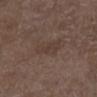Assessment:
Imaged during a routine full-body skin examination; the lesion was not biopsied and no histopathology is available.
Context:
The lesion is located on the leg. A male subject, roughly 75 years of age. This is a white-light tile. A region of skin cropped from a whole-body photographic capture, roughly 15 mm wide. The recorded lesion diameter is about 3.5 mm. The total-body-photography lesion software estimated an eccentricity of roughly 0.85 and a shape-asymmetry score of about 0.4 (0 = symmetric). The analysis additionally found a lesion color around L≈37 a*≈14 b*≈21 in CIELAB and a lesion–skin lightness drop of about 5. It also reported a border-irregularity rating of about 4.5/10 and a within-lesion color-variation index near 1/10.